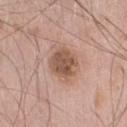{
  "lighting": "white-light",
  "site": "right lower leg",
  "image": {
    "source": "total-body photography crop",
    "field_of_view_mm": 15
  },
  "patient": {
    "sex": "male",
    "age_approx": 60
  },
  "lesion_size": {
    "long_diameter_mm_approx": 3.5
  }
}